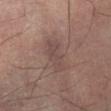notes: imaged on a skin check; not biopsied | illumination: cross-polarized illumination | lesion size: ≈5 mm | patient: male, aged around 50 | site: the left lower leg | image source: ~15 mm tile from a whole-body skin photo.